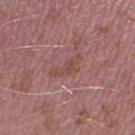Notes:
• lighting — white-light illumination
• location — the right lower leg
• TBP lesion metrics — a lesion area of about 5.5 mm² and a shape-asymmetry score of about 0.4 (0 = symmetric); a classifier nevus-likeness of about 0/100 and lesion-presence confidence of about 80/100
• subject — male, in their 40s
• image source — 15 mm crop, total-body photography
• lesion size — about 4 mm